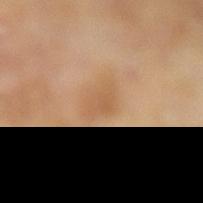notes = no biopsy performed (imaged during a skin exam)
patient = male, aged approximately 65
image = ~15 mm crop, total-body skin-cancer survey
illumination = cross-polarized illumination
lesion diameter = ~3.5 mm (longest diameter)
location = the leg